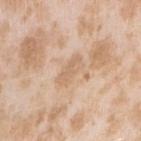Assessment: The lesion was tiled from a total-body skin photograph and was not biopsied. Acquisition and patient details: Located on the right upper arm. The subject is a female in their mid- to late 20s. A close-up tile cropped from a whole-body skin photograph, about 15 mm across.